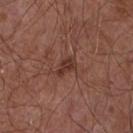Captured during whole-body skin photography for melanoma surveillance; the lesion was not biopsied. A male patient, approximately 55 years of age. Captured under white-light illumination. A 15 mm close-up extracted from a 3D total-body photography capture. Located on the chest. The lesion-visualizer software estimated a footprint of about 3.5 mm², an outline eccentricity of about 0.8 (0 = round, 1 = elongated), and two-axis asymmetry of about 0.4. It also reported an average lesion color of about L≈34 a*≈22 b*≈24 (CIELAB), about 9 CIELAB-L* units darker than the surrounding skin, and a normalized border contrast of about 8. Approximately 2.5 mm at its widest.